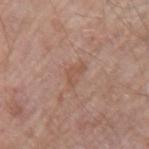Recorded during total-body skin imaging; not selected for excision or biopsy. The recorded lesion diameter is about 2.5 mm. A region of skin cropped from a whole-body photographic capture, roughly 15 mm wide. A male subject, aged around 65. This is a white-light tile. On the left upper arm.